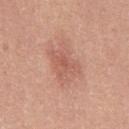{"biopsy_status": "not biopsied; imaged during a skin examination", "site": "mid back", "lesion_size": {"long_diameter_mm_approx": 5.0}, "image": {"source": "total-body photography crop", "field_of_view_mm": 15}, "lighting": "white-light", "automated_metrics": {"area_mm2_approx": 13.0, "eccentricity": 0.75, "border_irregularity_0_10": 3.5, "color_variation_0_10": 3.0, "peripheral_color_asymmetry": 1.0, "nevus_likeness_0_100": 0, "lesion_detection_confidence_0_100": 100}, "patient": {"sex": "male", "age_approx": 45}}